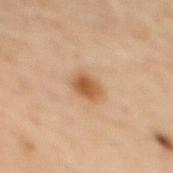Q: Was a biopsy performed?
A: imaged on a skin check; not biopsied
Q: What did automated image analysis measure?
A: a lesion area of about 5.5 mm², an outline eccentricity of about 0.65 (0 = round, 1 = elongated), and two-axis asymmetry of about 0.15; a border-irregularity rating of about 1.5/10, a within-lesion color-variation index near 4/10, and peripheral color asymmetry of about 1.5; lesion-presence confidence of about 100/100
Q: What kind of image is this?
A: 15 mm crop, total-body photography
Q: How large is the lesion?
A: ~3 mm (longest diameter)
Q: Patient demographics?
A: male, in their mid- to late 60s
Q: How was the tile lit?
A: cross-polarized illumination
Q: What is the anatomic site?
A: the mid back